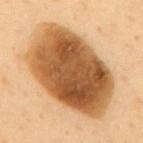On the mid back. A female subject, in their 50s. The total-body-photography lesion software estimated an eccentricity of roughly 0.8 and a symmetry-axis asymmetry near 0.1. The analysis additionally found a mean CIELAB color near L≈55 a*≈22 b*≈40 and a lesion-to-skin contrast of about 13 (normalized; higher = more distinct). The software also gave a border-irregularity index near 1.5/10 and a peripheral color-asymmetry measure near 2.5. And it measured a classifier nevus-likeness of about 35/100 and lesion-presence confidence of about 100/100. A 15 mm close-up extracted from a 3D total-body photography capture.